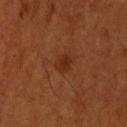biopsy_status: not biopsied; imaged during a skin examination
lesion_size:
  long_diameter_mm_approx: 2.5
automated_metrics:
  border_irregularity_0_10: 2.5
  color_variation_0_10: 3.0
  peripheral_color_asymmetry: 1.0
image:
  source: total-body photography crop
  field_of_view_mm: 15
site: upper back
lighting: cross-polarized
patient:
  sex: male
  age_approx: 60